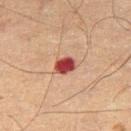Impression: No biopsy was performed on this lesion — it was imaged during a full skin examination and was not determined to be concerning. Acquisition and patient details: A male patient in their mid- to late 60s. Cropped from a whole-body photographic skin survey; the tile spans about 15 mm. From the right thigh. Imaged with cross-polarized lighting.